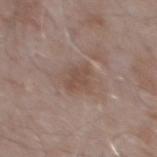Assessment:
The lesion was tiled from a total-body skin photograph and was not biopsied.
Image and clinical context:
A 15 mm close-up tile from a total-body photography series done for melanoma screening. From the mid back. The lesion's longest dimension is about 3 mm. The patient is a male about 55 years old.